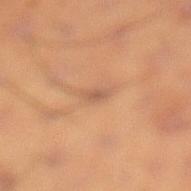Context: The recorded lesion diameter is about 2.5 mm. A 15 mm close-up extracted from a 3D total-body photography capture. On the leg. Imaged with cross-polarized lighting. The lesion-visualizer software estimated an outline eccentricity of about 0.9 (0 = round, 1 = elongated) and a symmetry-axis asymmetry near 0.2. And it measured a lesion color around L≈49 a*≈18 b*≈29 in CIELAB, about 7 CIELAB-L* units darker than the surrounding skin, and a lesion-to-skin contrast of about 6 (normalized; higher = more distinct). The software also gave a border-irregularity index near 3/10, a within-lesion color-variation index near 0/10, and peripheral color asymmetry of about 0. A male patient in their mid-50s.